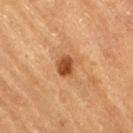Recorded during total-body skin imaging; not selected for excision or biopsy. A 15 mm close-up tile from a total-body photography series done for melanoma screening. The patient is a male aged approximately 85 or older. Automated image analysis of the tile measured an average lesion color of about L≈43 a*≈23 b*≈35 (CIELAB) and a normalized border contrast of about 9.5. The analysis additionally found border irregularity of about 1.5 on a 0–10 scale, a color-variation rating of about 3.5/10, and a peripheral color-asymmetry measure near 1. The lesion is located on the lower back. The tile uses cross-polarized illumination. Longest diameter approximately 2.5 mm.